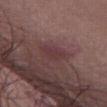A male subject approximately 70 years of age. On the front of the torso. A lesion tile, about 15 mm wide, cut from a 3D total-body photograph.